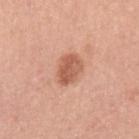Imaged during a routine full-body skin examination; the lesion was not biopsied and no histopathology is available.
Captured under white-light illumination.
Automated tile analysis of the lesion measured a lesion–skin lightness drop of about 12 and a lesion-to-skin contrast of about 7.5 (normalized; higher = more distinct). The analysis additionally found internal color variation of about 4 on a 0–10 scale and radial color variation of about 1.5.
Longest diameter approximately 3.5 mm.
From the left upper arm.
Cropped from a whole-body photographic skin survey; the tile spans about 15 mm.
The patient is a female approximately 35 years of age.